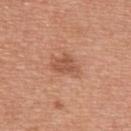{"biopsy_status": "not biopsied; imaged during a skin examination", "patient": {"sex": "male", "age_approx": 55}, "image": {"source": "total-body photography crop", "field_of_view_mm": 15}, "site": "back", "lighting": "white-light", "automated_metrics": {"area_mm2_approx": 6.5, "shape_asymmetry": 0.35, "vs_skin_darker_L": 9.0, "vs_skin_contrast_norm": 6.0}, "lesion_size": {"long_diameter_mm_approx": 3.5}}